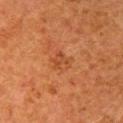The lesion was photographed on a routine skin check and not biopsied; there is no pathology result.
The recorded lesion diameter is about 3 mm.
The tile uses cross-polarized illumination.
From the right upper arm.
A female patient aged approximately 50.
A 15 mm close-up tile from a total-body photography series done for melanoma screening.